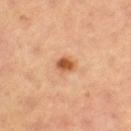A close-up tile cropped from a whole-body skin photograph, about 15 mm across. The recorded lesion diameter is about 2.5 mm. The total-body-photography lesion software estimated an area of roughly 4.5 mm², an outline eccentricity of about 0.45 (0 = round, 1 = elongated), and a shape-asymmetry score of about 0.25 (0 = symmetric). It also reported border irregularity of about 2 on a 0–10 scale, a color-variation rating of about 3.5/10, and a peripheral color-asymmetry measure near 1. It also reported a nevus-likeness score of about 95/100 and a lesion-detection confidence of about 100/100. The patient is a female aged 63 to 67. Located on the left thigh.